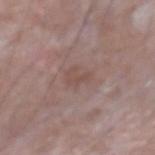Q: Lesion location?
A: the right upper arm
Q: How was the tile lit?
A: white-light
Q: Patient demographics?
A: male, about 65 years old
Q: Lesion size?
A: ~3 mm (longest diameter)
Q: What did automated image analysis measure?
A: an automated nevus-likeness rating near 0 out of 100 and a lesion-detection confidence of about 100/100
Q: What is the imaging modality?
A: total-body-photography crop, ~15 mm field of view